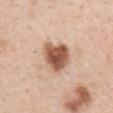  biopsy_status: not biopsied; imaged during a skin examination
  site: chest
  image:
    source: total-body photography crop
    field_of_view_mm: 15
  automated_metrics:
    eccentricity: 0.35
    shape_asymmetry: 0.3
    vs_skin_darker_L: 18.0
    vs_skin_contrast_norm: 11.0
    border_irregularity_0_10: 2.5
    peripheral_color_asymmetry: 2.0
    nevus_likeness_0_100: 95
  lesion_size:
    long_diameter_mm_approx: 4.0
  patient:
    sex: female
    age_approx: 30
  lighting: white-light